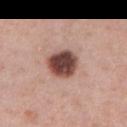  biopsy_status: not biopsied; imaged during a skin examination
  lighting: white-light
  site: chest
  lesion_size:
    long_diameter_mm_approx: 4.0
  patient:
    sex: male
    age_approx: 60
  automated_metrics:
    cielab_L: 46
    cielab_a: 22
    cielab_b: 23
    vs_skin_contrast_norm: 13.5
    color_variation_0_10: 6.0
    nevus_likeness_0_100: 45
    lesion_detection_confidence_0_100: 100
  image:
    source: total-body photography crop
    field_of_view_mm: 15